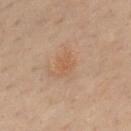Notes:
• follow-up — no biopsy performed (imaged during a skin exam)
• patient — male, roughly 50 years of age
• anatomic site — the mid back
• image — ~15 mm crop, total-body skin-cancer survey
• illumination — cross-polarized
• size — about 2.5 mm